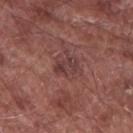The patient is a male roughly 75 years of age.
From the arm.
A roughly 15 mm field-of-view crop from a total-body skin photograph.
The lesion's longest dimension is about 2.5 mm.
The lesion-visualizer software estimated about 7 CIELAB-L* units darker than the surrounding skin and a normalized border contrast of about 6.5. The analysis additionally found a border-irregularity index near 2.5/10, internal color variation of about 3 on a 0–10 scale, and peripheral color asymmetry of about 1. The software also gave a classifier nevus-likeness of about 10/100 and a lesion-detection confidence of about 100/100.